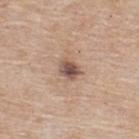No biopsy was performed on this lesion — it was imaged during a full skin examination and was not determined to be concerning.
This image is a 15 mm lesion crop taken from a total-body photograph.
From the back.
The subject is a male approximately 55 years of age.
The recorded lesion diameter is about 2.5 mm.
This is a white-light tile.